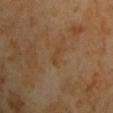Imaged during a routine full-body skin examination; the lesion was not biopsied and no histopathology is available.
A 15 mm close-up extracted from a 3D total-body photography capture.
Longest diameter approximately 2.5 mm.
The subject is a male aged approximately 65.
Captured under cross-polarized illumination.